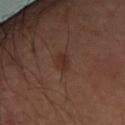Clinical impression:
No biopsy was performed on this lesion — it was imaged during a full skin examination and was not determined to be concerning.
Context:
From the left arm. A male subject approximately 50 years of age. A region of skin cropped from a whole-body photographic capture, roughly 15 mm wide. Measured at roughly 2.5 mm in maximum diameter.